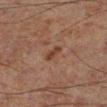This lesion was catalogued during total-body skin photography and was not selected for biopsy. The tile uses cross-polarized illumination. A male subject, in their 60s. On the left leg. About 2.5 mm across. A lesion tile, about 15 mm wide, cut from a 3D total-body photograph.